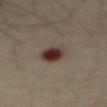workup = no biopsy performed (imaged during a skin exam) | body site = the abdomen | subject = male, aged approximately 60 | illumination = cross-polarized illumination | image = ~15 mm crop, total-body skin-cancer survey | automated lesion analysis = a footprint of about 8.5 mm², a shape eccentricity near 0.8, and two-axis asymmetry of about 0.2; an average lesion color of about L≈34 a*≈16 b*≈18 (CIELAB) and a lesion–skin lightness drop of about 13; a within-lesion color-variation index near 9/10 and peripheral color asymmetry of about 2.5; an automated nevus-likeness rating near 5 out of 100 and lesion-presence confidence of about 100/100.